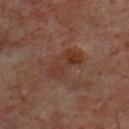| key | value |
|---|---|
| workup | no biopsy performed (imaged during a skin exam) |
| subject | male, in their 70s |
| anatomic site | the chest |
| image | ~15 mm crop, total-body skin-cancer survey |